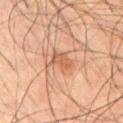The lesion-visualizer software estimated a mean CIELAB color near L≈55 a*≈21 b*≈32, a lesion–skin lightness drop of about 9, and a normalized border contrast of about 6. It also reported a classifier nevus-likeness of about 0/100.
The lesion is on the abdomen.
Cropped from a whole-body photographic skin survey; the tile spans about 15 mm.
The tile uses cross-polarized illumination.
A male subject approximately 55 years of age.